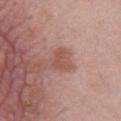| key | value |
|---|---|
| workup | imaged on a skin check; not biopsied |
| diameter | ~3 mm (longest diameter) |
| patient | male, about 50 years old |
| acquisition | ~15 mm crop, total-body skin-cancer survey |
| tile lighting | white-light |
| body site | the chest |
| automated metrics | a footprint of about 4.5 mm² and a shape-asymmetry score of about 0.35 (0 = symmetric); a border-irregularity index near 3/10, internal color variation of about 1.5 on a 0–10 scale, and radial color variation of about 0.5; a classifier nevus-likeness of about 10/100 and a detector confidence of about 100 out of 100 that the crop contains a lesion |